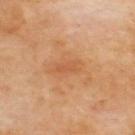Q: Was this lesion biopsied?
A: no biopsy performed (imaged during a skin exam)
Q: Where on the body is the lesion?
A: the back
Q: Automated lesion metrics?
A: border irregularity of about 2.5 on a 0–10 scale, internal color variation of about 1.5 on a 0–10 scale, and radial color variation of about 0.5
Q: What is the lesion's diameter?
A: ≈2.5 mm
Q: How was this image acquired?
A: total-body-photography crop, ~15 mm field of view
Q: Illumination type?
A: cross-polarized